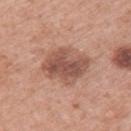image source = 15 mm crop, total-body photography
body site = the left upper arm
patient = female, aged around 50